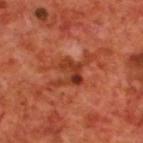Captured under cross-polarized illumination. The lesion is located on the upper back. The recorded lesion diameter is about 6 mm. A close-up tile cropped from a whole-body skin photograph, about 15 mm across. The subject is a male about 70 years old.